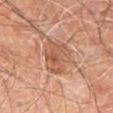This lesion was catalogued during total-body skin photography and was not selected for biopsy.